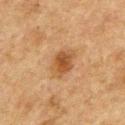| key | value |
|---|---|
| notes | catalogued during a skin exam; not biopsied |
| image | 15 mm crop, total-body photography |
| anatomic site | the chest |
| subject | male, roughly 75 years of age |